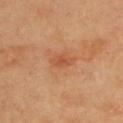Clinical impression:
The lesion was tiled from a total-body skin photograph and was not biopsied.
Background:
The lesion is on the upper back. A female subject roughly 60 years of age. A 15 mm crop from a total-body photograph taken for skin-cancer surveillance.